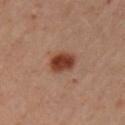follow-up: total-body-photography surveillance lesion; no biopsy
imaging modality: ~15 mm tile from a whole-body skin photo
diameter: ~3.5 mm (longest diameter)
TBP lesion metrics: an outline eccentricity of about 0.65 (0 = round, 1 = elongated) and a shape-asymmetry score of about 0.2 (0 = symmetric); a peripheral color-asymmetry measure near 1.5; an automated nevus-likeness rating near 100 out of 100 and a detector confidence of about 100 out of 100 that the crop contains a lesion
location: the arm
lighting: cross-polarized
patient: female, aged 43–47